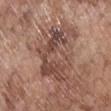This lesion was catalogued during total-body skin photography and was not selected for biopsy. Measured at roughly 6.5 mm in maximum diameter. A male patient aged around 70. A 15 mm crop from a total-body photograph taken for skin-cancer surveillance. On the chest.